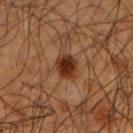Clinical impression: No biopsy was performed on this lesion — it was imaged during a full skin examination and was not determined to be concerning. Clinical summary: The patient is a male about 50 years old. Located on the left upper arm. A roughly 15 mm field-of-view crop from a total-body skin photograph.